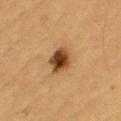Clinical impression:
The lesion was tiled from a total-body skin photograph and was not biopsied.
Image and clinical context:
A 15 mm close-up tile from a total-body photography series done for melanoma screening. The tile uses cross-polarized illumination. A male subject aged around 85 or older. The lesion-visualizer software estimated a lesion color around L≈38 a*≈19 b*≈33 in CIELAB, about 16 CIELAB-L* units darker than the surrounding skin, and a normalized lesion–skin contrast near 12.5. The analysis additionally found an automated nevus-likeness rating near 100 out of 100. Located on the arm.